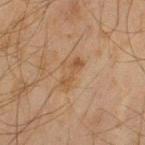Notes:
– follow-up · imaged on a skin check; not biopsied
– subject · male, roughly 45 years of age
– lighting · cross-polarized illumination
– anatomic site · the left thigh
– image source · ~15 mm tile from a whole-body skin photo
– image-analysis metrics · a footprint of about 4 mm², an eccentricity of roughly 0.95, and two-axis asymmetry of about 0.4; a mean CIELAB color near L≈42 a*≈16 b*≈29 and a normalized lesion–skin contrast near 6; border irregularity of about 5.5 on a 0–10 scale and internal color variation of about 1 on a 0–10 scale; an automated nevus-likeness rating near 0 out of 100 and a detector confidence of about 100 out of 100 that the crop contains a lesion